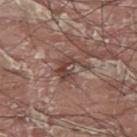No biopsy was performed on this lesion — it was imaged during a full skin examination and was not determined to be concerning.
On the upper back.
Imaged with white-light lighting.
Approximately 4 mm at its widest.
A male patient aged 38–42.
Cropped from a total-body skin-imaging series; the visible field is about 15 mm.